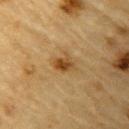| feature | finding |
|---|---|
| notes | no biopsy performed (imaged during a skin exam) |
| subject | male, aged 83 or older |
| image source | total-body-photography crop, ~15 mm field of view |
| site | the arm |